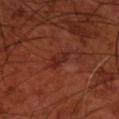{"biopsy_status": "not biopsied; imaged during a skin examination", "image": {"source": "total-body photography crop", "field_of_view_mm": 15}, "patient": {"sex": "male", "age_approx": 70}, "lesion_size": {"long_diameter_mm_approx": 3.5}, "site": "front of the torso", "lighting": "cross-polarized"}